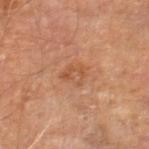The lesion was photographed on a routine skin check and not biopsied; there is no pathology result.
A roughly 15 mm field-of-view crop from a total-body skin photograph.
Approximately 2.5 mm at its widest.
The tile uses cross-polarized illumination.
A male patient, about 70 years old.
Automated image analysis of the tile measured a shape eccentricity near 0.5 and two-axis asymmetry of about 0.3. The analysis additionally found a lesion-to-skin contrast of about 5 (normalized; higher = more distinct). The software also gave border irregularity of about 3 on a 0–10 scale, internal color variation of about 4.5 on a 0–10 scale, and peripheral color asymmetry of about 1.5.
The lesion is located on the right thigh.